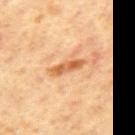Part of a total-body skin-imaging series; this lesion was reviewed on a skin check and was not flagged for biopsy. The tile uses cross-polarized illumination. A close-up tile cropped from a whole-body skin photograph, about 15 mm across. Approximately 4 mm at its widest. A male patient, aged approximately 65. The lesion is located on the mid back.